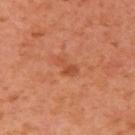illumination: cross-polarized | lesion size: ≈3 mm | automated metrics: a lesion area of about 3.5 mm², a shape eccentricity near 0.85, and two-axis asymmetry of about 0.4; a lesion–skin lightness drop of about 8 and a lesion-to-skin contrast of about 6 (normalized; higher = more distinct); a border-irregularity index near 4.5/10, a color-variation rating of about 1/10, and peripheral color asymmetry of about 0.5; a nevus-likeness score of about 0/100 and a lesion-detection confidence of about 100/100 | patient: female, aged 38 to 42 | location: the right upper arm | image: 15 mm crop, total-body photography.